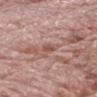{"biopsy_status": "not biopsied; imaged during a skin examination", "site": "head or neck", "patient": {"sex": "male", "age_approx": 70}, "automated_metrics": {"border_irregularity_0_10": 3.0, "color_variation_0_10": 1.0, "nevus_likeness_0_100": 0, "lesion_detection_confidence_0_100": 65}, "lesion_size": {"long_diameter_mm_approx": 2.5}, "image": {"source": "total-body photography crop", "field_of_view_mm": 15}}